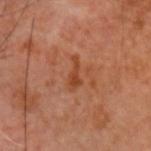Captured during whole-body skin photography for melanoma surveillance; the lesion was not biopsied. The recorded lesion diameter is about 3.5 mm. This image is a 15 mm lesion crop taken from a total-body photograph. A male subject aged 58–62. The lesion is on the head or neck. Captured under cross-polarized illumination.